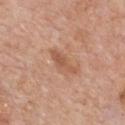notes: total-body-photography surveillance lesion; no biopsy
TBP lesion metrics: a border-irregularity index near 4.5/10 and a within-lesion color-variation index near 1/10; a detector confidence of about 100 out of 100 that the crop contains a lesion
imaging modality: ~15 mm tile from a whole-body skin photo
diameter: about 4 mm
lighting: white-light illumination
location: the chest
subject: male, roughly 60 years of age